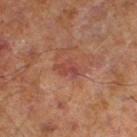{
  "biopsy_status": "not biopsied; imaged during a skin examination",
  "image": {
    "source": "total-body photography crop",
    "field_of_view_mm": 15
  },
  "lesion_size": {
    "long_diameter_mm_approx": 3.0
  },
  "site": "left lower leg",
  "patient": {
    "sex": "male",
    "age_approx": 70
  }
}